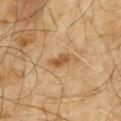Q: Is there a histopathology result?
A: total-body-photography surveillance lesion; no biopsy
Q: Lesion location?
A: the mid back
Q: Patient demographics?
A: male, roughly 65 years of age
Q: What kind of image is this?
A: total-body-photography crop, ~15 mm field of view